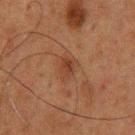biopsy status: imaged on a skin check; not biopsied | location: the upper back | size: ≈2.5 mm | imaging modality: ~15 mm crop, total-body skin-cancer survey | patient: male, aged 58–62.